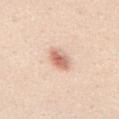Imaged during a routine full-body skin examination; the lesion was not biopsied and no histopathology is available. A roughly 15 mm field-of-view crop from a total-body skin photograph. This is a white-light tile. The recorded lesion diameter is about 3 mm. The subject is a male in their mid-20s. On the back.